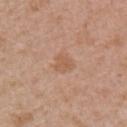The lesion was tiled from a total-body skin photograph and was not biopsied. Approximately 2.5 mm at its widest. A 15 mm close-up extracted from a 3D total-body photography capture. A female patient, roughly 60 years of age. The lesion is on the right upper arm.